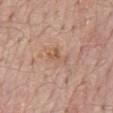| key | value |
|---|---|
| notes | catalogued during a skin exam; not biopsied |
| location | the mid back |
| subject | male, aged 68 to 72 |
| tile lighting | white-light |
| lesion diameter | about 3 mm |
| image source | ~15 mm crop, total-body skin-cancer survey |